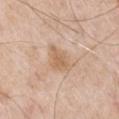Findings:
* tile lighting · white-light
* lesion size · ≈4 mm
* acquisition · ~15 mm tile from a whole-body skin photo
* anatomic site · the arm
* patient · male, in their mid- to late 60s